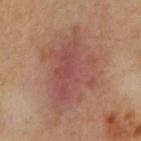| key | value |
|---|---|
| lesion size | about 8.5 mm |
| imaging modality | ~15 mm crop, total-body skin-cancer survey |
| tile lighting | cross-polarized |
| patient | male, aged 63 to 67 |
| anatomic site | the abdomen |